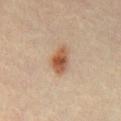workup: total-body-photography surveillance lesion; no biopsy | patient: male, about 35 years old | location: the chest | automated metrics: a lesion–skin lightness drop of about 12 and a lesion-to-skin contrast of about 9 (normalized; higher = more distinct); a classifier nevus-likeness of about 100/100 and a lesion-detection confidence of about 100/100 | lesion diameter: ≈4 mm | acquisition: ~15 mm crop, total-body skin-cancer survey | illumination: cross-polarized illumination.